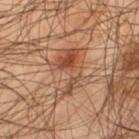* follow-up: imaged on a skin check; not biopsied
* patient: male, aged 53 to 57
* tile lighting: cross-polarized
* image source: ~15 mm crop, total-body skin-cancer survey
* lesion size: ~6 mm (longest diameter)
* site: the left thigh